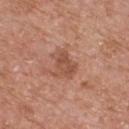Q: Is there a histopathology result?
A: no biopsy performed (imaged during a skin exam)
Q: What lighting was used for the tile?
A: white-light illumination
Q: What is the anatomic site?
A: the upper back
Q: Patient demographics?
A: male, in their mid-70s
Q: What did automated image analysis measure?
A: a lesion color around L≈51 a*≈24 b*≈31 in CIELAB and roughly 9 lightness units darker than nearby skin; a nevus-likeness score of about 0/100 and a lesion-detection confidence of about 100/100
Q: What is the lesion's diameter?
A: about 3.5 mm
Q: What kind of image is this?
A: 15 mm crop, total-body photography